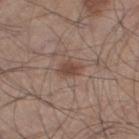Notes:
– notes · imaged on a skin check; not biopsied
– patient · male, approximately 45 years of age
– image source · total-body-photography crop, ~15 mm field of view
– location · the right thigh